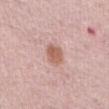Findings:
– notes — imaged on a skin check; not biopsied
– image — ~15 mm tile from a whole-body skin photo
– location — the abdomen
– patient — female, roughly 65 years of age
– automated lesion analysis — a color-variation rating of about 2.5/10; an automated nevus-likeness rating near 95 out of 100
– illumination — white-light